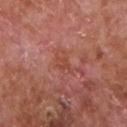Case summary:
* workup: total-body-photography surveillance lesion; no biopsy
* body site: the chest
* illumination: white-light
* subject: male, aged 63–67
* lesion size: ≈3.5 mm
* automated metrics: an eccentricity of roughly 0.85; a border-irregularity rating of about 3/10 and a peripheral color-asymmetry measure near 0.5; an automated nevus-likeness rating near 0 out of 100 and lesion-presence confidence of about 100/100
* image: ~15 mm crop, total-body skin-cancer survey